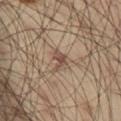A male subject, aged 38–42. The lesion is on the left thigh. Imaged with cross-polarized lighting. Cropped from a whole-body photographic skin survey; the tile spans about 15 mm. The recorded lesion diameter is about 3 mm.